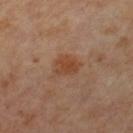biopsy status — catalogued during a skin exam; not biopsied
anatomic site — the left upper arm
imaging modality — ~15 mm tile from a whole-body skin photo
subject — female, aged around 55